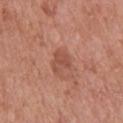Q: Is there a histopathology result?
A: no biopsy performed (imaged during a skin exam)
Q: What kind of image is this?
A: ~15 mm tile from a whole-body skin photo
Q: What are the patient's age and sex?
A: male, aged approximately 80
Q: Lesion location?
A: the upper back